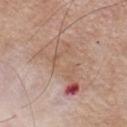The lesion is on the chest.
The total-body-photography lesion software estimated a footprint of about 20 mm², an eccentricity of roughly 0.9, and a shape-asymmetry score of about 0.55 (0 = symmetric). The analysis additionally found an average lesion color of about L≈58 a*≈19 b*≈27 (CIELAB), a lesion–skin lightness drop of about 8, and a normalized lesion–skin contrast near 5.5. The software also gave border irregularity of about 7.5 on a 0–10 scale, a color-variation rating of about 7/10, and a peripheral color-asymmetry measure near 1.5.
A close-up tile cropped from a whole-body skin photograph, about 15 mm across.
This is a white-light tile.
The recorded lesion diameter is about 7.5 mm.
The patient is a male aged approximately 55.